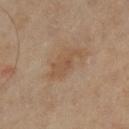Part of a total-body skin-imaging series; this lesion was reviewed on a skin check and was not flagged for biopsy. The lesion's longest dimension is about 4.5 mm. Imaged with cross-polarized lighting. A female subject aged around 70. Cropped from a total-body skin-imaging series; the visible field is about 15 mm. From the leg.